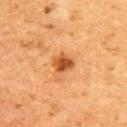| key | value |
|---|---|
| diameter | ~2.5 mm (longest diameter) |
| lighting | cross-polarized |
| anatomic site | the upper back |
| subject | female, aged 58 to 62 |
| image-analysis metrics | peripheral color asymmetry of about 1 |
| image | ~15 mm crop, total-body skin-cancer survey |